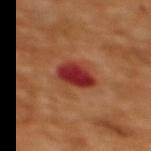Findings:
* acquisition: ~15 mm crop, total-body skin-cancer survey
* body site: the upper back
* lesion diameter: about 4 mm
* patient: female, approximately 55 years of age